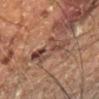This lesion was catalogued during total-body skin photography and was not selected for biopsy. The patient is a male aged 58 to 62. A 15 mm close-up tile from a total-body photography series done for melanoma screening. On the right leg.